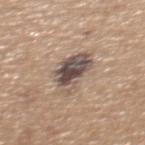notes: imaged on a skin check; not biopsied | illumination: white-light | anatomic site: the upper back | image-analysis metrics: a border-irregularity index near 4/10 and internal color variation of about 8.5 on a 0–10 scale; an automated nevus-likeness rating near 65 out of 100 | patient: male, aged 63–67 | image: 15 mm crop, total-body photography.